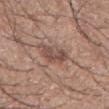Findings:
• workup: no biopsy performed (imaged during a skin exam)
• acquisition: ~15 mm crop, total-body skin-cancer survey
• tile lighting: white-light illumination
• location: the right forearm
• image-analysis metrics: a lesion area of about 7.5 mm² and a shape-asymmetry score of about 0.25 (0 = symmetric); a lesion color around L≈49 a*≈19 b*≈24 in CIELAB, about 10 CIELAB-L* units darker than the surrounding skin, and a normalized border contrast of about 7; border irregularity of about 3 on a 0–10 scale, a within-lesion color-variation index near 4/10, and radial color variation of about 1; a classifier nevus-likeness of about 10/100 and a lesion-detection confidence of about 95/100
• diameter: ≈3.5 mm
• subject: male, about 65 years old